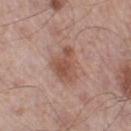Imaged during a routine full-body skin examination; the lesion was not biopsied and no histopathology is available. A region of skin cropped from a whole-body photographic capture, roughly 15 mm wide. The lesion is on the left thigh. The recorded lesion diameter is about 4 mm. A male patient in their mid- to late 50s. Imaged with white-light lighting.